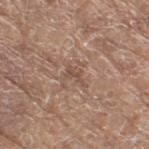Impression: This lesion was catalogued during total-body skin photography and was not selected for biopsy. Background: The lesion is located on the right thigh. A roughly 15 mm field-of-view crop from a total-body skin photograph. About 2.5 mm across. The patient is a female aged around 75.